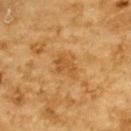follow-up = catalogued during a skin exam; not biopsied
location = the upper back
diameter = ≈3.5 mm
imaging modality = ~15 mm tile from a whole-body skin photo
lighting = cross-polarized
subject = male, about 85 years old or older